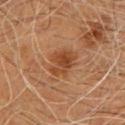The total-body-photography lesion software estimated a footprint of about 8 mm², a shape eccentricity near 0.65, and two-axis asymmetry of about 0.25. It also reported a lesion color around L≈41 a*≈22 b*≈34 in CIELAB, a lesion–skin lightness drop of about 9, and a normalized lesion–skin contrast near 7.5. The software also gave a nevus-likeness score of about 75/100 and lesion-presence confidence of about 100/100. A male patient aged around 60. Located on the chest. A 15 mm close-up tile from a total-body photography series done for melanoma screening. Approximately 4 mm at its widest.